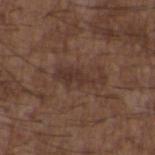Background:
Automated image analysis of the tile measured an area of roughly 7.5 mm², an eccentricity of roughly 0.95, and two-axis asymmetry of about 0.55. It also reported a mean CIELAB color near L≈33 a*≈17 b*≈22, a lesion–skin lightness drop of about 6, and a normalized border contrast of about 6.5. The software also gave border irregularity of about 8.5 on a 0–10 scale, a color-variation rating of about 1.5/10, and radial color variation of about 0.5. On the back. Longest diameter approximately 5.5 mm. The patient is a male aged 48–52. A 15 mm close-up tile from a total-body photography series done for melanoma screening. This is a white-light tile.